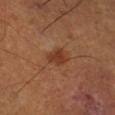No biopsy was performed on this lesion — it was imaged during a full skin examination and was not determined to be concerning.
The tile uses cross-polarized illumination.
The lesion is located on the right lower leg.
The lesion-visualizer software estimated an average lesion color of about L≈37 a*≈24 b*≈32 (CIELAB), about 8 CIELAB-L* units darker than the surrounding skin, and a normalized border contrast of about 7.5. The software also gave a nevus-likeness score of about 60/100.
A male patient, approximately 50 years of age.
A lesion tile, about 15 mm wide, cut from a 3D total-body photograph.
Longest diameter approximately 2.5 mm.